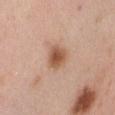Clinical impression:
This lesion was catalogued during total-body skin photography and was not selected for biopsy.